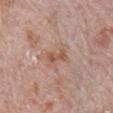biopsy_status: not biopsied; imaged during a skin examination
patient:
  sex: male
  age_approx: 70
site: right upper arm
image:
  source: total-body photography crop
  field_of_view_mm: 15
lesion_size:
  long_diameter_mm_approx: 3.0